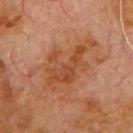<tbp_lesion>
<biopsy_status>not biopsied; imaged during a skin examination</biopsy_status>
<lighting>cross-polarized</lighting>
<image>
  <source>total-body photography crop</source>
  <field_of_view_mm>15</field_of_view_mm>
</image>
<patient>
  <sex>male</sex>
  <age_approx>80</age_approx>
</patient>
<site>front of the torso</site>
<lesion_size>
  <long_diameter_mm_approx>8.0</long_diameter_mm_approx>
</lesion_size>
</tbp_lesion>